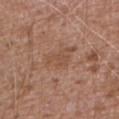biopsy_status: not biopsied; imaged during a skin examination